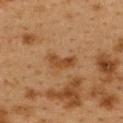image = 15 mm crop, total-body photography
subject = female, aged 38–42
lesion size = ~3.5 mm (longest diameter)
illumination = cross-polarized illumination
site = the upper back